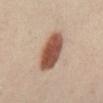Q: Was a biopsy performed?
A: no biopsy performed (imaged during a skin exam)
Q: Who is the patient?
A: female, aged approximately 40
Q: What kind of image is this?
A: ~15 mm tile from a whole-body skin photo
Q: Where on the body is the lesion?
A: the left leg
Q: How large is the lesion?
A: ~5 mm (longest diameter)
Q: What lighting was used for the tile?
A: cross-polarized